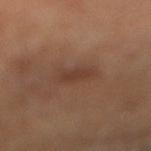  biopsy_status: not biopsied; imaged during a skin examination